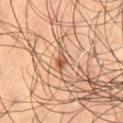Clinical impression: Part of a total-body skin-imaging series; this lesion was reviewed on a skin check and was not flagged for biopsy. Clinical summary: A 15 mm crop from a total-body photograph taken for skin-cancer surveillance. The patient is a male in their mid- to late 60s. Imaged with cross-polarized lighting. The lesion is on the right thigh. The recorded lesion diameter is about 2 mm.